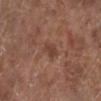Q: Is there a histopathology result?
A: total-body-photography surveillance lesion; no biopsy